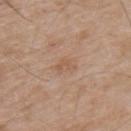This lesion was catalogued during total-body skin photography and was not selected for biopsy. The lesion is located on the upper back. The patient is a male about 55 years old. A 15 mm close-up extracted from a 3D total-body photography capture.